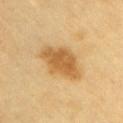No biopsy was performed on this lesion — it was imaged during a full skin examination and was not determined to be concerning. A male subject, aged approximately 60. The lesion is located on the chest. The recorded lesion diameter is about 6 mm. A 15 mm close-up extracted from a 3D total-body photography capture.